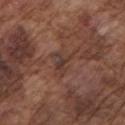Case summary:
* biopsy status — catalogued during a skin exam; not biopsied
* tile lighting — white-light
* anatomic site — the left upper arm
* patient — male, in their mid- to late 70s
* image source — ~15 mm tile from a whole-body skin photo